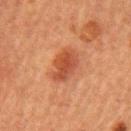Case summary:
* follow-up: catalogued during a skin exam; not biopsied
* site: the left upper arm
* subject: female, approximately 55 years of age
* tile lighting: cross-polarized illumination
* image source: ~15 mm tile from a whole-body skin photo
* automated lesion analysis: a mean CIELAB color near L≈40 a*≈26 b*≈31, roughly 9 lightness units darker than nearby skin, and a normalized border contrast of about 7.5; a nevus-likeness score of about 95/100 and a detector confidence of about 100 out of 100 that the crop contains a lesion